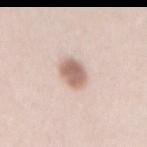  biopsy_status: not biopsied; imaged during a skin examination
  site: lower back
  patient:
    sex: female
    age_approx: 45
  lesion_size:
    long_diameter_mm_approx: 3.5
  image:
    source: total-body photography crop
    field_of_view_mm: 15
  lighting: white-light